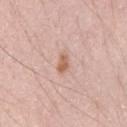Image and clinical context:
From the left upper arm. A close-up tile cropped from a whole-body skin photograph, about 15 mm across. The lesion's longest dimension is about 2.5 mm. Captured under white-light illumination. A male subject about 25 years old.